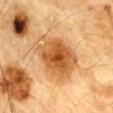| feature | finding |
|---|---|
| biopsy status | no biopsy performed (imaged during a skin exam) |
| patient | male, roughly 85 years of age or older |
| automated metrics | a lesion area of about 24 mm², an eccentricity of roughly 0.7, and two-axis asymmetry of about 0.25; border irregularity of about 2.5 on a 0–10 scale and internal color variation of about 7 on a 0–10 scale; an automated nevus-likeness rating near 5 out of 100 and a lesion-detection confidence of about 100/100 |
| lesion diameter | ≈6.5 mm |
| imaging modality | total-body-photography crop, ~15 mm field of view |
| anatomic site | the chest |
| tile lighting | cross-polarized |